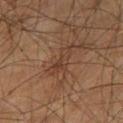Impression:
This lesion was catalogued during total-body skin photography and was not selected for biopsy.
Context:
The patient is a male in their 60s. Approximately 4 mm at its widest. A lesion tile, about 15 mm wide, cut from a 3D total-body photograph. The lesion is on the left thigh.